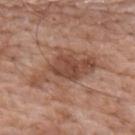biopsy_status: not biopsied; imaged during a skin examination
patient:
  sex: male
  age_approx: 60
automated_metrics:
  area_mm2_approx: 19.0
  eccentricity: 0.9
lighting: white-light
image:
  source: total-body photography crop
  field_of_view_mm: 15
site: mid back